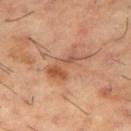{"biopsy_status": "not biopsied; imaged during a skin examination", "image": {"source": "total-body photography crop", "field_of_view_mm": 15}, "patient": {"sex": "male", "age_approx": 65}, "site": "right thigh"}